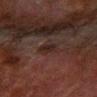No biopsy was performed on this lesion — it was imaged during a full skin examination and was not determined to be concerning. The patient is a male about 80 years old. Approximately 2.5 mm at its widest. A region of skin cropped from a whole-body photographic capture, roughly 15 mm wide. Located on the left forearm. Automated tile analysis of the lesion measured an area of roughly 4.5 mm², an eccentricity of roughly 0.55, and a shape-asymmetry score of about 0.15 (0 = symmetric). It also reported a mean CIELAB color near L≈18 a*≈13 b*≈15 and a normalized border contrast of about 6.5. The software also gave a border-irregularity rating of about 2.5/10, a within-lesion color-variation index near 1.5/10, and a peripheral color-asymmetry measure near 0.5. It also reported a classifier nevus-likeness of about 20/100. The tile uses cross-polarized illumination.